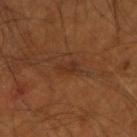The lesion was photographed on a routine skin check and not biopsied; there is no pathology result.
A roughly 15 mm field-of-view crop from a total-body skin photograph.
About 3 mm across.
A male subject roughly 55 years of age.
Captured under cross-polarized illumination.
The lesion is on the right forearm.